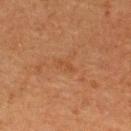Clinical impression: The lesion was photographed on a routine skin check and not biopsied; there is no pathology result. Image and clinical context: From the back. A roughly 15 mm field-of-view crop from a total-body skin photograph. A female patient, about 40 years old.